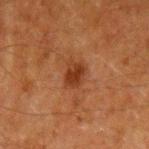This lesion was catalogued during total-body skin photography and was not selected for biopsy.
Captured under cross-polarized illumination.
From the left upper arm.
The recorded lesion diameter is about 3 mm.
The total-body-photography lesion software estimated a classifier nevus-likeness of about 80/100 and a detector confidence of about 100 out of 100 that the crop contains a lesion.
A male patient about 60 years old.
A lesion tile, about 15 mm wide, cut from a 3D total-body photograph.